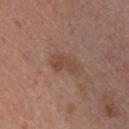No biopsy was performed on this lesion — it was imaged during a full skin examination and was not determined to be concerning. From the front of the torso. Approximately 3.5 mm at its widest. Automated image analysis of the tile measured a border-irregularity index near 3/10 and a color-variation rating of about 2.5/10. Imaged with white-light lighting. A male patient about 25 years old. A lesion tile, about 15 mm wide, cut from a 3D total-body photograph.